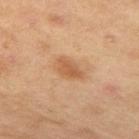Recorded during total-body skin imaging; not selected for excision or biopsy. Automated image analysis of the tile measured about 8 CIELAB-L* units darker than the surrounding skin and a normalized border contrast of about 6.5. And it measured border irregularity of about 3 on a 0–10 scale and a peripheral color-asymmetry measure near 1. A region of skin cropped from a whole-body photographic capture, roughly 15 mm wide. The lesion is on the upper back. The patient is a female in their 60s.